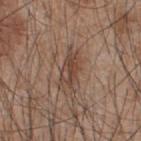Captured under white-light illumination. The lesion is located on the upper back. The recorded lesion diameter is about 5 mm. The total-body-photography lesion software estimated a footprint of about 8 mm², an eccentricity of roughly 0.9, and a shape-asymmetry score of about 0.3 (0 = symmetric). The analysis additionally found a nevus-likeness score of about 10/100 and a lesion-detection confidence of about 95/100. A close-up tile cropped from a whole-body skin photograph, about 15 mm across. A male patient in their mid-40s.